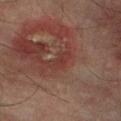No biopsy was performed on this lesion — it was imaged during a full skin examination and was not determined to be concerning.
The recorded lesion diameter is about 3 mm.
The subject is a male aged around 75.
A region of skin cropped from a whole-body photographic capture, roughly 15 mm wide.
An algorithmic analysis of the crop reported an area of roughly 3.5 mm², an outline eccentricity of about 0.8 (0 = round, 1 = elongated), and a symmetry-axis asymmetry near 0.45. It also reported a mean CIELAB color near L≈30 a*≈21 b*≈20. The software also gave an automated nevus-likeness rating near 0 out of 100.
The tile uses cross-polarized illumination.
Located on the left lower leg.